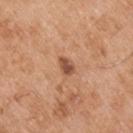The lesion was tiled from a total-body skin photograph and was not biopsied. An algorithmic analysis of the crop reported an area of roughly 3.5 mm² and an eccentricity of roughly 0.75. The analysis additionally found a nevus-likeness score of about 40/100 and a lesion-detection confidence of about 100/100. The lesion is on the right upper arm. Imaged with white-light lighting. A male patient, aged around 55. The recorded lesion diameter is about 2.5 mm. A 15 mm close-up extracted from a 3D total-body photography capture.